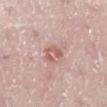Q: Is there a histopathology result?
A: no biopsy performed (imaged during a skin exam)
Q: How was this image acquired?
A: ~15 mm crop, total-body skin-cancer survey
Q: What are the patient's age and sex?
A: female, aged around 25
Q: Where on the body is the lesion?
A: the right lower leg